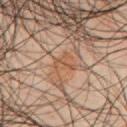workup = total-body-photography surveillance lesion; no biopsy | patient = male, roughly 50 years of age | acquisition = ~15 mm tile from a whole-body skin photo | tile lighting = cross-polarized illumination | lesion diameter = about 2.5 mm | site = the chest.